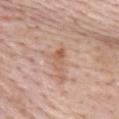<record>
  <biopsy_status>not biopsied; imaged during a skin examination</biopsy_status>
  <patient>
    <sex>female</sex>
    <age_approx>70</age_approx>
  </patient>
  <site>upper back</site>
  <lighting>white-light</lighting>
  <image>
    <source>total-body photography crop</source>
    <field_of_view_mm>15</field_of_view_mm>
  </image>
</record>